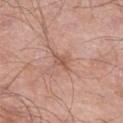Part of a total-body skin-imaging series; this lesion was reviewed on a skin check and was not flagged for biopsy.
A 15 mm close-up extracted from a 3D total-body photography capture.
A male patient, aged 68 to 72.
The lesion is on the leg.